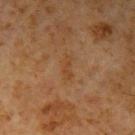Captured under cross-polarized illumination. From the arm. A 15 mm close-up extracted from a 3D total-body photography capture. The recorded lesion diameter is about 2.5 mm. The subject is a male in their 60s. Automated tile analysis of the lesion measured a shape eccentricity near 0.9 and a shape-asymmetry score of about 0.35 (0 = symmetric). It also reported a lesion color around L≈35 a*≈17 b*≈30 in CIELAB, about 5 CIELAB-L* units darker than the surrounding skin, and a normalized border contrast of about 5. The software also gave a nevus-likeness score of about 0/100 and a lesion-detection confidence of about 100/100.